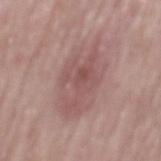* follow-up — no biopsy performed (imaged during a skin exam)
* TBP lesion metrics — a mean CIELAB color near L≈53 a*≈21 b*≈20 and a normalized border contrast of about 6; a border-irregularity index near 3/10, a within-lesion color-variation index near 4/10, and peripheral color asymmetry of about 1.5; a lesion-detection confidence of about 100/100
* site — the back
* lighting — white-light illumination
* image — ~15 mm crop, total-body skin-cancer survey
* subject — male, in their mid- to late 60s